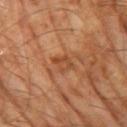<tbp_lesion>
<image>
  <source>total-body photography crop</source>
  <field_of_view_mm>15</field_of_view_mm>
</image>
<lesion_size>
  <long_diameter_mm_approx>2.5</long_diameter_mm_approx>
</lesion_size>
<patient>
  <sex>male</sex>
  <age_approx>65</age_approx>
</patient>
<lighting>cross-polarized</lighting>
</tbp_lesion>